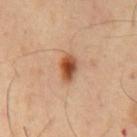Captured during whole-body skin photography for melanoma surveillance; the lesion was not biopsied. Measured at roughly 3.5 mm in maximum diameter. Automated image analysis of the tile measured a mean CIELAB color near L≈50 a*≈24 b*≈35, a lesion–skin lightness drop of about 15, and a lesion-to-skin contrast of about 11 (normalized; higher = more distinct). The software also gave an automated nevus-likeness rating near 100 out of 100 and a detector confidence of about 100 out of 100 that the crop contains a lesion. From the chest. A male subject, roughly 55 years of age. Captured under cross-polarized illumination. This image is a 15 mm lesion crop taken from a total-body photograph.